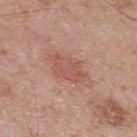Part of a total-body skin-imaging series; this lesion was reviewed on a skin check and was not flagged for biopsy.
Located on the upper back.
The lesion's longest dimension is about 5 mm.
A male patient, aged 68–72.
Cropped from a whole-body photographic skin survey; the tile spans about 15 mm.